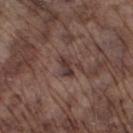Part of a total-body skin-imaging series; this lesion was reviewed on a skin check and was not flagged for biopsy. The lesion's longest dimension is about 3 mm. Imaged with white-light lighting. The lesion is on the left lower leg. A male subject, aged approximately 75. Cropped from a whole-body photographic skin survey; the tile spans about 15 mm.